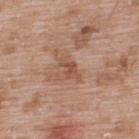Q: Is there a histopathology result?
A: imaged on a skin check; not biopsied
Q: How was the tile lit?
A: white-light illumination
Q: Automated lesion metrics?
A: an area of roughly 3 mm² and an outline eccentricity of about 0.85 (0 = round, 1 = elongated)
Q: What are the patient's age and sex?
A: male, about 50 years old
Q: What kind of image is this?
A: 15 mm crop, total-body photography
Q: What is the anatomic site?
A: the upper back